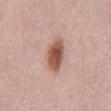| key | value |
|---|---|
| notes | no biopsy performed (imaged during a skin exam) |
| patient | male, aged approximately 50 |
| site | the abdomen |
| lighting | white-light |
| automated lesion analysis | a lesion area of about 9 mm², a shape eccentricity near 0.85, and a shape-asymmetry score of about 0.15 (0 = symmetric); a mean CIELAB color near L≈55 a*≈23 b*≈28, roughly 15 lightness units darker than nearby skin, and a normalized lesion–skin contrast near 10; a nevus-likeness score of about 100/100 and lesion-presence confidence of about 100/100 |
| acquisition | ~15 mm crop, total-body skin-cancer survey |
| lesion size | ~4.5 mm (longest diameter) |